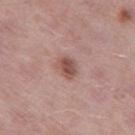<record>
  <biopsy_status>not biopsied; imaged during a skin examination</biopsy_status>
  <site>leg</site>
  <patient>
    <sex>male</sex>
    <age_approx>55</age_approx>
  </patient>
  <lesion_size>
    <long_diameter_mm_approx>2.5</long_diameter_mm_approx>
  </lesion_size>
  <image>
    <source>total-body photography crop</source>
    <field_of_view_mm>15</field_of_view_mm>
  </image>
  <automated_metrics>
    <area_mm2_approx>4.5</area_mm2_approx>
    <cielab_L>51</cielab_L>
    <cielab_a>22</cielab_a>
    <cielab_b>24</cielab_b>
    <vs_skin_darker_L>12.0</vs_skin_darker_L>
    <vs_skin_contrast_norm>8.5</vs_skin_contrast_norm>
    <border_irregularity_0_10>2.0</border_irregularity_0_10>
    <color_variation_0_10>3.5</color_variation_0_10>
    <peripheral_color_asymmetry>1.5</peripheral_color_asymmetry>
    <nevus_likeness_0_100>85</nevus_likeness_0_100>
    <lesion_detection_confidence_0_100>100</lesion_detection_confidence_0_100>
  </automated_metrics>
  <lighting>white-light</lighting>
</record>